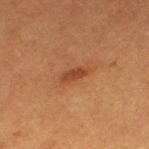Captured during whole-body skin photography for melanoma surveillance; the lesion was not biopsied.
A female subject in their 40s.
Captured under cross-polarized illumination.
Measured at roughly 3 mm in maximum diameter.
On the leg.
Cropped from a whole-body photographic skin survey; the tile spans about 15 mm.